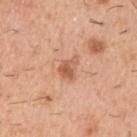Case summary:
– workup — total-body-photography surveillance lesion; no biopsy
– TBP lesion metrics — a footprint of about 4.5 mm² and a shape-asymmetry score of about 0.4 (0 = symmetric); about 11 CIELAB-L* units darker than the surrounding skin and a normalized border contrast of about 7; a within-lesion color-variation index near 4/10 and a peripheral color-asymmetry measure near 1.5
– size — ~3 mm (longest diameter)
– anatomic site — the arm
– subject — male, aged 38 to 42
– imaging modality — ~15 mm tile from a whole-body skin photo